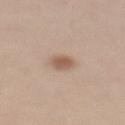workup=catalogued during a skin exam; not biopsied
diameter=~2.5 mm (longest diameter)
body site=the mid back
tile lighting=white-light illumination
automated lesion analysis=a lesion area of about 4 mm², an eccentricity of roughly 0.75, and two-axis asymmetry of about 0.2; a border-irregularity index near 2/10 and radial color variation of about 0.5; a classifier nevus-likeness of about 95/100
image=~15 mm tile from a whole-body skin photo
patient=female, roughly 65 years of age